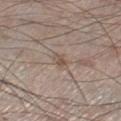follow-up = no biopsy performed (imaged during a skin exam); tile lighting = white-light; diameter = ~2.5 mm (longest diameter); subject = male, about 60 years old; site = the right lower leg; imaging modality = ~15 mm crop, total-body skin-cancer survey.